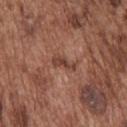Imaged with white-light lighting. Cropped from a total-body skin-imaging series; the visible field is about 15 mm. The lesion is located on the upper back. The subject is a male approximately 75 years of age. An algorithmic analysis of the crop reported a shape eccentricity near 0.95 and two-axis asymmetry of about 0.45. And it measured a lesion color around L≈42 a*≈23 b*≈27 in CIELAB, about 9 CIELAB-L* units darker than the surrounding skin, and a normalized border contrast of about 7.5. It also reported border irregularity of about 5.5 on a 0–10 scale, a color-variation rating of about 0.5/10, and peripheral color asymmetry of about 0. The software also gave an automated nevus-likeness rating near 0 out of 100.